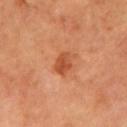{
  "biopsy_status": "not biopsied; imaged during a skin examination",
  "site": "left upper arm",
  "lesion_size": {
    "long_diameter_mm_approx": 3.0
  },
  "image": {
    "source": "total-body photography crop",
    "field_of_view_mm": 15
  },
  "patient": {
    "sex": "male",
    "age_approx": 65
  }
}